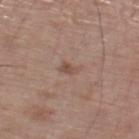  biopsy_status: not biopsied; imaged during a skin examination
  image:
    source: total-body photography crop
    field_of_view_mm: 15
  lesion_size:
    long_diameter_mm_approx: 2.5
  site: right thigh
  patient:
    sex: male
    age_approx: 70